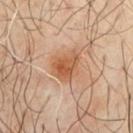Clinical impression: Recorded during total-body skin imaging; not selected for excision or biopsy. Image and clinical context: A lesion tile, about 15 mm wide, cut from a 3D total-body photograph. A male patient, aged 63–67. Imaged with cross-polarized lighting. An algorithmic analysis of the crop reported an eccentricity of roughly 0.5 and a symmetry-axis asymmetry near 0.25. On the chest.